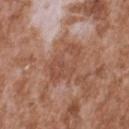The lesion was tiled from a total-body skin photograph and was not biopsied.
Automated image analysis of the tile measured a border-irregularity index near 7.5/10, internal color variation of about 2.5 on a 0–10 scale, and a peripheral color-asymmetry measure near 0.5.
This is a white-light tile.
Cropped from a total-body skin-imaging series; the visible field is about 15 mm.
The lesion is located on the upper back.
About 5 mm across.
A male subject, aged approximately 45.